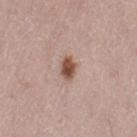Recorded during total-body skin imaging; not selected for excision or biopsy.
On the left thigh.
Imaged with white-light lighting.
The patient is a female in their 50s.
Cropped from a total-body skin-imaging series; the visible field is about 15 mm.
The recorded lesion diameter is about 2.5 mm.